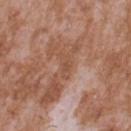Assessment: The lesion was photographed on a routine skin check and not biopsied; there is no pathology result. Clinical summary: The patient is a male about 45 years old. This is a white-light tile. A lesion tile, about 15 mm wide, cut from a 3D total-body photograph. From the upper back.